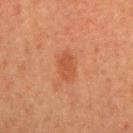Q: Was a biopsy performed?
A: catalogued during a skin exam; not biopsied
Q: What kind of image is this?
A: total-body-photography crop, ~15 mm field of view
Q: What lighting was used for the tile?
A: cross-polarized illumination
Q: What did automated image analysis measure?
A: a footprint of about 5.5 mm² and a shape eccentricity near 0.8
Q: Where on the body is the lesion?
A: the right upper arm
Q: Who is the patient?
A: female, aged 53–57